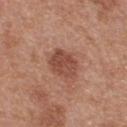Part of a total-body skin-imaging series; this lesion was reviewed on a skin check and was not flagged for biopsy.
The recorded lesion diameter is about 3.5 mm.
Located on the upper back.
A 15 mm close-up tile from a total-body photography series done for melanoma screening.
The tile uses white-light illumination.
Automated image analysis of the tile measured an average lesion color of about L≈47 a*≈24 b*≈28 (CIELAB), about 11 CIELAB-L* units darker than the surrounding skin, and a lesion-to-skin contrast of about 7.5 (normalized; higher = more distinct). The analysis additionally found a border-irregularity index near 2/10 and a color-variation rating of about 3.5/10. The software also gave a classifier nevus-likeness of about 80/100 and a detector confidence of about 100 out of 100 that the crop contains a lesion.
A male subject, aged 28 to 32.